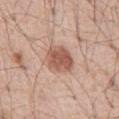No biopsy was performed on this lesion — it was imaged during a full skin examination and was not determined to be concerning. From the abdomen. Approximately 4 mm at its widest. Automated image analysis of the tile measured a lesion area of about 9 mm². The software also gave an average lesion color of about L≈56 a*≈23 b*≈28 (CIELAB), about 13 CIELAB-L* units darker than the surrounding skin, and a lesion-to-skin contrast of about 8.5 (normalized; higher = more distinct). The software also gave border irregularity of about 1.5 on a 0–10 scale, internal color variation of about 3 on a 0–10 scale, and radial color variation of about 1. A roughly 15 mm field-of-view crop from a total-body skin photograph. This is a white-light tile. The subject is a male aged 53 to 57.